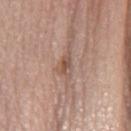  biopsy_status: not biopsied; imaged during a skin examination
  image:
    source: total-body photography crop
    field_of_view_mm: 15
  patient:
    sex: female
    age_approx: 70
  site: left forearm
  lighting: white-light
  lesion_size:
    long_diameter_mm_approx: 2.5